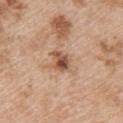follow-up = total-body-photography surveillance lesion; no biopsy | illumination = white-light | body site = the upper back | subject = female, aged 43 to 47 | image = 15 mm crop, total-body photography | TBP lesion metrics = about 13 CIELAB-L* units darker than the surrounding skin and a normalized border contrast of about 9; border irregularity of about 3 on a 0–10 scale and radial color variation of about 3.